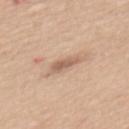Part of a total-body skin-imaging series; this lesion was reviewed on a skin check and was not flagged for biopsy. The lesion is located on the mid back. A female subject, roughly 55 years of age. The lesion's longest dimension is about 3 mm. Cropped from a total-body skin-imaging series; the visible field is about 15 mm. Imaged with white-light lighting.